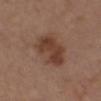Clinical summary:
A female patient, in their 40s. A region of skin cropped from a whole-body photographic capture, roughly 15 mm wide. On the chest.